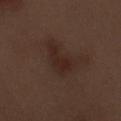biopsy status: no biopsy performed (imaged during a skin exam)
lesion size: ≈4.5 mm
subject: male, aged 68 to 72
acquisition: 15 mm crop, total-body photography
body site: the left forearm
lighting: white-light illumination